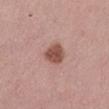follow-up = imaged on a skin check; not biopsied | lesion size = ~3 mm (longest diameter) | illumination = white-light | patient = female, approximately 45 years of age | imaging modality = ~15 mm crop, total-body skin-cancer survey | location = the left thigh | automated lesion analysis = an area of roughly 6.5 mm², a shape eccentricity near 0.65, and a symmetry-axis asymmetry near 0.25; a lesion color around L≈51 a*≈23 b*≈25 in CIELAB, a lesion–skin lightness drop of about 13, and a normalized lesion–skin contrast near 9.5; a classifier nevus-likeness of about 95/100.